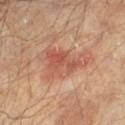<tbp_lesion>
  <biopsy_status>not biopsied; imaged during a skin examination</biopsy_status>
  <lesion_size>
    <long_diameter_mm_approx>5.5</long_diameter_mm_approx>
  </lesion_size>
  <patient>
    <sex>male</sex>
    <age_approx>65</age_approx>
  </patient>
  <site>left lower leg</site>
  <image>
    <source>total-body photography crop</source>
    <field_of_view_mm>15</field_of_view_mm>
  </image>
</tbp_lesion>